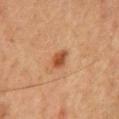Assessment:
The lesion was tiled from a total-body skin photograph and was not biopsied.
Acquisition and patient details:
From the chest. A 15 mm crop from a total-body photograph taken for skin-cancer surveillance. This is a cross-polarized tile. The patient is a male aged approximately 50. The total-body-photography lesion software estimated a border-irregularity rating of about 2/10, a color-variation rating of about 2/10, and a peripheral color-asymmetry measure near 0.5. The software also gave a nevus-likeness score of about 90/100.